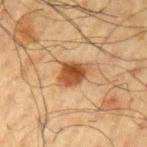biopsy status: imaged on a skin check; not biopsied | acquisition: 15 mm crop, total-body photography | body site: the left upper arm | patient: male, aged around 60.